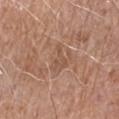Q: Patient demographics?
A: male, in their 70s
Q: What is the imaging modality?
A: ~15 mm crop, total-body skin-cancer survey
Q: Lesion location?
A: the right forearm